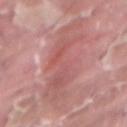Assessment: The lesion was tiled from a total-body skin photograph and was not biopsied. Acquisition and patient details: The patient is a male roughly 40 years of age. The lesion's longest dimension is about 10.5 mm. The tile uses white-light illumination. A close-up tile cropped from a whole-body skin photograph, about 15 mm across. Located on the abdomen.